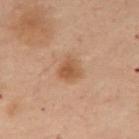The lesion was photographed on a routine skin check and not biopsied; there is no pathology result. A female subject aged 53–57. The lesion is located on the chest. Imaged with cross-polarized lighting. An algorithmic analysis of the crop reported an average lesion color of about L≈45 a*≈18 b*≈30 (CIELAB) and a normalized lesion–skin contrast near 7. The software also gave an automated nevus-likeness rating near 80 out of 100 and a detector confidence of about 100 out of 100 that the crop contains a lesion. Longest diameter approximately 3 mm. A 15 mm close-up extracted from a 3D total-body photography capture.